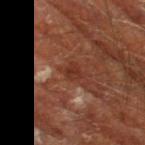No biopsy was performed on this lesion — it was imaged during a full skin examination and was not determined to be concerning. The total-body-photography lesion software estimated an area of roughly 4 mm², a shape eccentricity near 0.75, and two-axis asymmetry of about 0.2. A 15 mm close-up extracted from a 3D total-body photography capture. Imaged with cross-polarized lighting. The patient is a male roughly 65 years of age. The lesion is located on the leg.